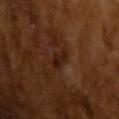This lesion was catalogued during total-body skin photography and was not selected for biopsy.
A region of skin cropped from a whole-body photographic capture, roughly 15 mm wide.
Captured under cross-polarized illumination.
Automated tile analysis of the lesion measured an area of roughly 3.5 mm² and a symmetry-axis asymmetry near 0.3. The software also gave a classifier nevus-likeness of about 15/100 and a detector confidence of about 100 out of 100 that the crop contains a lesion.
The subject is a male roughly 65 years of age.
Measured at roughly 3 mm in maximum diameter.